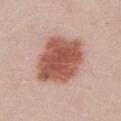Clinical impression: No biopsy was performed on this lesion — it was imaged during a full skin examination and was not determined to be concerning. Background: Captured under white-light illumination. The lesion's longest dimension is about 6.5 mm. On the left upper arm. The total-body-photography lesion software estimated a lesion area of about 27 mm², a shape eccentricity near 0.6, and a shape-asymmetry score of about 0.15 (0 = symmetric). The analysis additionally found a mean CIELAB color near L≈54 a*≈25 b*≈28, about 16 CIELAB-L* units darker than the surrounding skin, and a lesion-to-skin contrast of about 10.5 (normalized; higher = more distinct). The analysis additionally found border irregularity of about 2 on a 0–10 scale, a within-lesion color-variation index near 4.5/10, and radial color variation of about 1.5. And it measured a classifier nevus-likeness of about 100/100 and lesion-presence confidence of about 100/100. A 15 mm close-up tile from a total-body photography series done for melanoma screening. A male patient, approximately 25 years of age.